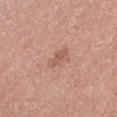No biopsy was performed on this lesion — it was imaged during a full skin examination and was not determined to be concerning. This is a white-light tile. A female patient, approximately 50 years of age. A close-up tile cropped from a whole-body skin photograph, about 15 mm across. Approximately 3 mm at its widest. The lesion is located on the left lower leg.